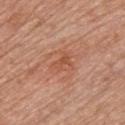notes = total-body-photography surveillance lesion; no biopsy
image-analysis metrics = a border-irregularity index near 4.5/10 and a color-variation rating of about 3/10
illumination = white-light illumination
body site = the chest
image source = ~15 mm tile from a whole-body skin photo
size = ~2.5 mm (longest diameter)
subject = male, approximately 60 years of age